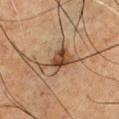Background:
Automated image analysis of the tile measured a footprint of about 8.5 mm², an outline eccentricity of about 0.75 (0 = round, 1 = elongated), and a shape-asymmetry score of about 0.55 (0 = symmetric). It also reported an average lesion color of about L≈36 a*≈15 b*≈27 (CIELAB) and a normalized lesion–skin contrast near 9. The analysis additionally found a border-irregularity index near 6.5/10, internal color variation of about 5.5 on a 0–10 scale, and peripheral color asymmetry of about 2.5. The software also gave a classifier nevus-likeness of about 85/100 and lesion-presence confidence of about 100/100. On the chest. A male subject, about 50 years old. Captured under cross-polarized illumination. Cropped from a whole-body photographic skin survey; the tile spans about 15 mm.